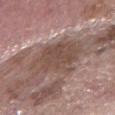Context:
Located on the right forearm. Cropped from a whole-body photographic skin survey; the tile spans about 15 mm. This is a white-light tile. Longest diameter approximately 5.5 mm. A male subject, about 60 years old.